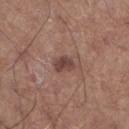Q: Is there a histopathology result?
A: no biopsy performed (imaged during a skin exam)
Q: What did automated image analysis measure?
A: an area of roughly 4 mm², a shape eccentricity near 0.75, and a symmetry-axis asymmetry near 0.25; a lesion color around L≈43 a*≈20 b*≈22 in CIELAB, about 11 CIELAB-L* units darker than the surrounding skin, and a normalized lesion–skin contrast near 8.5; a border-irregularity rating of about 2.5/10 and radial color variation of about 0.5
Q: What lighting was used for the tile?
A: white-light
Q: How large is the lesion?
A: ≈2.5 mm
Q: Where on the body is the lesion?
A: the left lower leg
Q: What are the patient's age and sex?
A: male, aged around 60
Q: What is the imaging modality?
A: total-body-photography crop, ~15 mm field of view